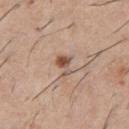follow-up = total-body-photography surveillance lesion; no biopsy
site = the front of the torso
patient = male, aged approximately 60
automated metrics = an average lesion color of about L≈53 a*≈19 b*≈28 (CIELAB), a lesion–skin lightness drop of about 12, and a normalized lesion–skin contrast near 8.5; a border-irregularity rating of about 5.5/10, a color-variation rating of about 2.5/10, and radial color variation of about 0.5
imaging modality = 15 mm crop, total-body photography
lighting = white-light illumination